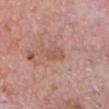Recorded during total-body skin imaging; not selected for excision or biopsy. Captured under white-light illumination. On the head or neck. Approximately 3 mm at its widest. The patient is a male roughly 60 years of age. A lesion tile, about 15 mm wide, cut from a 3D total-body photograph.